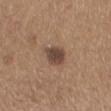<case>
<biopsy_status>not biopsied; imaged during a skin examination</biopsy_status>
<automated_metrics>
  <area_mm2_approx>6.0</area_mm2_approx>
  <eccentricity>0.65</eccentricity>
  <nevus_likeness_0_100>75</nevus_likeness_0_100>
  <lesion_detection_confidence_0_100>100</lesion_detection_confidence_0_100>
</automated_metrics>
<lesion_size>
  <long_diameter_mm_approx>3.5</long_diameter_mm_approx>
</lesion_size>
<patient>
  <sex>male</sex>
  <age_approx>65</age_approx>
</patient>
<image>
  <source>total-body photography crop</source>
  <field_of_view_mm>15</field_of_view_mm>
</image>
<lighting>white-light</lighting>
<site>mid back</site>
</case>